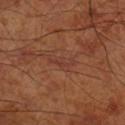Findings:
- workup — catalogued during a skin exam; not biopsied